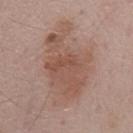No biopsy was performed on this lesion — it was imaged during a full skin examination and was not determined to be concerning. Located on the mid back. A male subject in their mid- to late 50s. This is a white-light tile. A lesion tile, about 15 mm wide, cut from a 3D total-body photograph. Longest diameter approximately 10 mm.